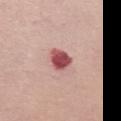Imaged during a routine full-body skin examination; the lesion was not biopsied and no histopathology is available.
Cropped from a total-body skin-imaging series; the visible field is about 15 mm.
The subject is a female aged around 60.
The lesion is on the mid back.